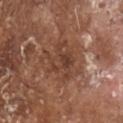Q: What are the patient's age and sex?
A: male, aged 78–82
Q: What is the imaging modality?
A: total-body-photography crop, ~15 mm field of view
Q: What is the anatomic site?
A: the head or neck